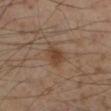Imaged during a routine full-body skin examination; the lesion was not biopsied and no histopathology is available. Approximately 3 mm at its widest. The lesion is located on the left lower leg. The subject is a male aged 53–57. A region of skin cropped from a whole-body photographic capture, roughly 15 mm wide. The tile uses cross-polarized illumination.